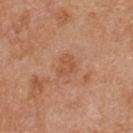Q: Was a biopsy performed?
A: catalogued during a skin exam; not biopsied
Q: How was this image acquired?
A: 15 mm crop, total-body photography
Q: What did automated image analysis measure?
A: a color-variation rating of about 2.5/10 and radial color variation of about 1; an automated nevus-likeness rating near 0 out of 100 and a lesion-detection confidence of about 100/100
Q: Lesion location?
A: the upper back
Q: Illumination type?
A: white-light illumination
Q: How large is the lesion?
A: about 2.5 mm
Q: Patient demographics?
A: female, aged around 40